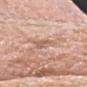biopsy status: total-body-photography surveillance lesion; no biopsy
subject: male, aged around 75
lighting: white-light
lesion size: ~3.5 mm (longest diameter)
anatomic site: the head or neck
imaging modality: ~15 mm tile from a whole-body skin photo